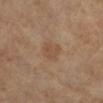Impression:
The lesion was photographed on a routine skin check and not biopsied; there is no pathology result.
Acquisition and patient details:
A female subject, roughly 70 years of age. A 15 mm close-up tile from a total-body photography series done for melanoma screening. The lesion is on the left lower leg.